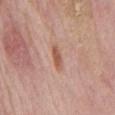The lesion was photographed on a routine skin check and not biopsied; there is no pathology result. A male patient, approximately 75 years of age. A 15 mm close-up tile from a total-body photography series done for melanoma screening. Imaged with white-light lighting. Longest diameter approximately 3 mm. On the back.